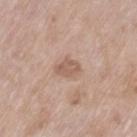body site = the left thigh; size = ~3 mm (longest diameter); acquisition = ~15 mm crop, total-body skin-cancer survey; patient = female, aged 73–77.